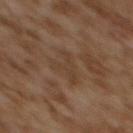| field | value |
|---|---|
| biopsy status | imaged on a skin check; not biopsied |
| acquisition | ~15 mm crop, total-body skin-cancer survey |
| subject | female, aged approximately 60 |
| site | the upper back |
| lesion size | about 3.5 mm |